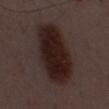Clinical impression: This lesion was catalogued during total-body skin photography and was not selected for biopsy. Context: A male patient, about 50 years old. The lesion-visualizer software estimated a classifier nevus-likeness of about 100/100 and a lesion-detection confidence of about 100/100. Imaged with white-light lighting. A 15 mm close-up tile from a total-body photography series done for melanoma screening.